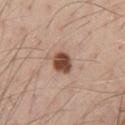No biopsy was performed on this lesion — it was imaged during a full skin examination and was not determined to be concerning. Automated tile analysis of the lesion measured a mean CIELAB color near L≈47 a*≈21 b*≈29, about 18 CIELAB-L* units darker than the surrounding skin, and a normalized border contrast of about 12.5. The analysis additionally found border irregularity of about 1 on a 0–10 scale, internal color variation of about 3.5 on a 0–10 scale, and peripheral color asymmetry of about 1. The analysis additionally found a classifier nevus-likeness of about 95/100 and a detector confidence of about 100 out of 100 that the crop contains a lesion. About 2.5 mm across. This is a white-light tile. On the front of the torso. A 15 mm close-up extracted from a 3D total-body photography capture. A male subject roughly 40 years of age.